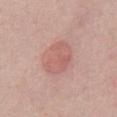Q: Is there a histopathology result?
A: no biopsy performed (imaged during a skin exam)
Q: What are the patient's age and sex?
A: male, roughly 40 years of age
Q: What is the imaging modality?
A: total-body-photography crop, ~15 mm field of view
Q: How was the tile lit?
A: white-light illumination
Q: Where on the body is the lesion?
A: the abdomen
Q: What is the lesion's diameter?
A: ~4.5 mm (longest diameter)